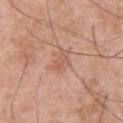Acquisition and patient details: A male patient, aged 53 to 57. The lesion is located on the back. A region of skin cropped from a whole-body photographic capture, roughly 15 mm wide.